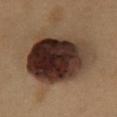workup — catalogued during a skin exam; not biopsied | subject — male, aged 48 to 52 | lesion diameter — ~8.5 mm (longest diameter) | location — the front of the torso | acquisition — total-body-photography crop, ~15 mm field of view.